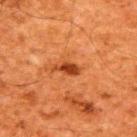Part of a total-body skin-imaging series; this lesion was reviewed on a skin check and was not flagged for biopsy.
A lesion tile, about 15 mm wide, cut from a 3D total-body photograph.
Automated tile analysis of the lesion measured a border-irregularity index near 3/10 and a color-variation rating of about 1/10. The software also gave lesion-presence confidence of about 100/100.
This is a cross-polarized tile.
Located on the upper back.
The subject is a male in their 60s.